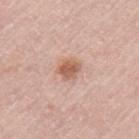Part of a total-body skin-imaging series; this lesion was reviewed on a skin check and was not flagged for biopsy. The tile uses white-light illumination. Longest diameter approximately 2.5 mm. The lesion-visualizer software estimated a shape-asymmetry score of about 0.3 (0 = symmetric). It also reported a lesion color around L≈59 a*≈22 b*≈30 in CIELAB, a lesion–skin lightness drop of about 12, and a normalized border contrast of about 8.5. The software also gave a border-irregularity index near 3/10, a within-lesion color-variation index near 2.5/10, and a peripheral color-asymmetry measure near 1. And it measured a nevus-likeness score of about 90/100. On the left upper arm. A 15 mm close-up tile from a total-body photography series done for melanoma screening. The subject is a female about 65 years old.